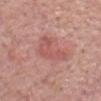Part of a total-body skin-imaging series; this lesion was reviewed on a skin check and was not flagged for biopsy.
Longest diameter approximately 5 mm.
On the head or neck.
The subject is a male about 75 years old.
Captured under white-light illumination.
An algorithmic analysis of the crop reported a lesion area of about 8.5 mm², an outline eccentricity of about 0.9 (0 = round, 1 = elongated), and two-axis asymmetry of about 0.6. The software also gave an average lesion color of about L≈55 a*≈27 b*≈24 (CIELAB), roughly 8 lightness units darker than nearby skin, and a normalized lesion–skin contrast near 5.5.
A 15 mm close-up extracted from a 3D total-body photography capture.